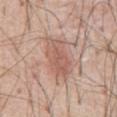{"biopsy_status": "not biopsied; imaged during a skin examination", "site": "abdomen", "lesion_size": {"long_diameter_mm_approx": 6.0}, "automated_metrics": {"cielab_L": 58, "cielab_a": 21, "cielab_b": 27, "vs_skin_darker_L": 9.0, "vs_skin_contrast_norm": 6.0, "nevus_likeness_0_100": 100, "lesion_detection_confidence_0_100": 100}, "patient": {"sex": "male", "age_approx": 55}, "image": {"source": "total-body photography crop", "field_of_view_mm": 15}, "lighting": "white-light"}